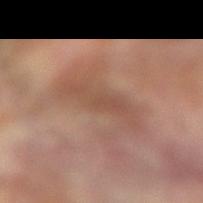Imaged during a routine full-body skin examination; the lesion was not biopsied and no histopathology is available. The subject is a female aged 73 to 77. Located on the right forearm. A region of skin cropped from a whole-body photographic capture, roughly 15 mm wide.